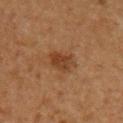Case summary:
- workup · no biopsy performed (imaged during a skin exam)
- subject · male, aged 63–67
- TBP lesion metrics · a mean CIELAB color near L≈33 a*≈19 b*≈29 and a normalized border contrast of about 7.5; lesion-presence confidence of about 100/100
- image source · ~15 mm tile from a whole-body skin photo
- diameter · about 3 mm
- body site · the front of the torso
- tile lighting · cross-polarized